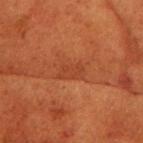workup: imaged on a skin check; not biopsied
lesion size: about 3 mm
subject: female, in their 80s
imaging modality: 15 mm crop, total-body photography
lighting: cross-polarized
image-analysis metrics: an average lesion color of about L≈32 a*≈24 b*≈30 (CIELAB) and a normalized border contrast of about 4.5; a border-irregularity rating of about 3/10, a within-lesion color-variation index near 1/10, and radial color variation of about 0.5; a nevus-likeness score of about 0/100 and a detector confidence of about 95 out of 100 that the crop contains a lesion
anatomic site: the head or neck